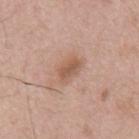{"biopsy_status": "not biopsied; imaged during a skin examination", "lighting": "white-light", "site": "mid back", "patient": {"sex": "male", "age_approx": 65}, "image": {"source": "total-body photography crop", "field_of_view_mm": 15}}